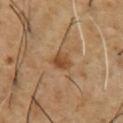Q: What kind of image is this?
A: 15 mm crop, total-body photography
Q: Lesion size?
A: about 2.5 mm
Q: Patient demographics?
A: male, aged 53 to 57
Q: Where on the body is the lesion?
A: the chest
Q: Automated lesion metrics?
A: a lesion area of about 4.5 mm² and an eccentricity of roughly 0.55; a classifier nevus-likeness of about 50/100
Q: Illumination type?
A: cross-polarized illumination